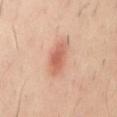Clinical impression:
Imaged during a routine full-body skin examination; the lesion was not biopsied and no histopathology is available.
Acquisition and patient details:
A region of skin cropped from a whole-body photographic capture, roughly 15 mm wide. The lesion is located on the back. This is a cross-polarized tile. The subject is a male aged around 40. The recorded lesion diameter is about 4.5 mm.